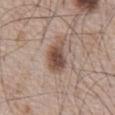biopsy status — total-body-photography surveillance lesion; no biopsy | body site — the chest | lighting — white-light | automated lesion analysis — a footprint of about 9 mm² and an eccentricity of roughly 0.85; a mean CIELAB color near L≈49 a*≈18 b*≈25, about 13 CIELAB-L* units darker than the surrounding skin, and a normalized border contrast of about 9.5 | imaging modality — ~15 mm tile from a whole-body skin photo | patient — male, about 65 years old.